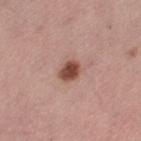The lesion-visualizer software estimated a lesion area of about 5 mm² and an eccentricity of roughly 0.55. It also reported a lesion-to-skin contrast of about 10.5 (normalized; higher = more distinct). It also reported internal color variation of about 3 on a 0–10 scale and a peripheral color-asymmetry measure near 1. The lesion's longest dimension is about 2.5 mm. From the left thigh. A region of skin cropped from a whole-body photographic capture, roughly 15 mm wide. A female patient, roughly 55 years of age. Imaged with white-light lighting.